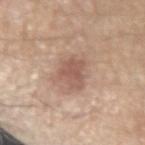Notes:
* follow-up — total-body-photography surveillance lesion; no biopsy
* imaging modality — total-body-photography crop, ~15 mm field of view
* size — ~4 mm (longest diameter)
* automated metrics — an average lesion color of about L≈56 a*≈19 b*≈26 (CIELAB); a nevus-likeness score of about 40/100
* subject — male, aged approximately 70
* location — the left forearm
* tile lighting — white-light illumination